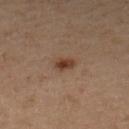notes=no biopsy performed (imaged during a skin exam) | size=~2.5 mm (longest diameter) | image=total-body-photography crop, ~15 mm field of view | patient=male, about 65 years old | site=the leg.